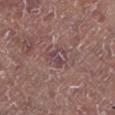Captured during whole-body skin photography for melanoma surveillance; the lesion was not biopsied.